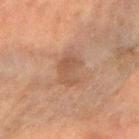Part of a total-body skin-imaging series; this lesion was reviewed on a skin check and was not flagged for biopsy. This image is a 15 mm lesion crop taken from a total-body photograph. The lesion is on the arm. Measured at roughly 4 mm in maximum diameter. The tile uses cross-polarized illumination. A female subject, roughly 60 years of age.